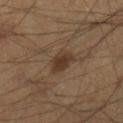Impression: The lesion was tiled from a total-body skin photograph and was not biopsied. Image and clinical context: The tile uses cross-polarized illumination. The lesion is located on the right lower leg. The total-body-photography lesion software estimated a footprint of about 6 mm², an eccentricity of roughly 0.7, and a shape-asymmetry score of about 0.2 (0 = symmetric). The software also gave a border-irregularity index near 2/10. Measured at roughly 3.5 mm in maximum diameter. The subject is a male aged 38–42. Cropped from a whole-body photographic skin survey; the tile spans about 15 mm.